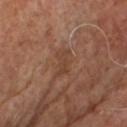Captured during whole-body skin photography for melanoma surveillance; the lesion was not biopsied.
A roughly 15 mm field-of-view crop from a total-body skin photograph.
Imaged with cross-polarized lighting.
About 3.5 mm across.
A male subject in their mid-60s.
The lesion is on the chest.
An algorithmic analysis of the crop reported an average lesion color of about L≈39 a*≈19 b*≈28 (CIELAB), about 6 CIELAB-L* units darker than the surrounding skin, and a normalized lesion–skin contrast near 5.5. The software also gave a border-irregularity rating of about 6/10, a within-lesion color-variation index near 0/10, and a peripheral color-asymmetry measure near 0. And it measured a nevus-likeness score of about 0/100.